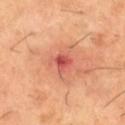illumination = cross-polarized illumination | body site = the left thigh | subject = male, approximately 60 years of age | acquisition = 15 mm crop, total-body photography | size = ≈3 mm.